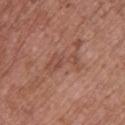Captured during whole-body skin photography for melanoma surveillance; the lesion was not biopsied. Cropped from a whole-body photographic skin survey; the tile spans about 15 mm. Captured under white-light illumination. The patient is a female aged 63 to 67. From the upper back. About 4.5 mm across. Automated tile analysis of the lesion measured a lesion color around L≈49 a*≈23 b*≈27 in CIELAB, about 7 CIELAB-L* units darker than the surrounding skin, and a normalized border contrast of about 5. The software also gave a nevus-likeness score of about 0/100 and lesion-presence confidence of about 100/100.